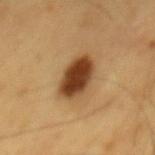biopsy status: no biopsy performed (imaged during a skin exam); site: the back; image: ~15 mm crop, total-body skin-cancer survey; subject: male, approximately 60 years of age.